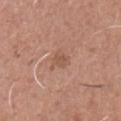Assessment:
This lesion was catalogued during total-body skin photography and was not selected for biopsy.
Image and clinical context:
A lesion tile, about 15 mm wide, cut from a 3D total-body photograph. The total-body-photography lesion software estimated an automated nevus-likeness rating near 0 out of 100. On the front of the torso. A male patient about 45 years old.